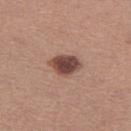Recorded during total-body skin imaging; not selected for excision or biopsy.
Cropped from a total-body skin-imaging series; the visible field is about 15 mm.
Measured at roughly 4 mm in maximum diameter.
A female subject aged around 35.
This is a white-light tile.
The lesion is on the right thigh.